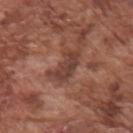No biopsy was performed on this lesion — it was imaged during a full skin examination and was not determined to be concerning.
Captured under white-light illumination.
A male patient aged 73 to 77.
A 15 mm close-up tile from a total-body photography series done for melanoma screening.
On the right upper arm.